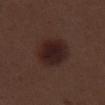biopsy status: catalogued during a skin exam; not biopsied
location: the right thigh
automated metrics: an eccentricity of roughly 0.55 and two-axis asymmetry of about 0.15; a border-irregularity index near 1.5/10, a color-variation rating of about 4/10, and a peripheral color-asymmetry measure near 1; a nevus-likeness score of about 95/100
image: ~15 mm crop, total-body skin-cancer survey
lighting: white-light illumination
size: ≈5 mm
patient: male, aged 68–72